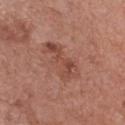Q: Is there a histopathology result?
A: total-body-photography surveillance lesion; no biopsy
Q: Who is the patient?
A: male, roughly 80 years of age
Q: What is the lesion's diameter?
A: about 5.5 mm
Q: Illumination type?
A: white-light
Q: Automated lesion metrics?
A: a lesion area of about 9 mm² and a shape eccentricity near 0.95; internal color variation of about 3.5 on a 0–10 scale and peripheral color asymmetry of about 1; an automated nevus-likeness rating near 10 out of 100
Q: What is the anatomic site?
A: the chest
Q: What kind of image is this?
A: 15 mm crop, total-body photography